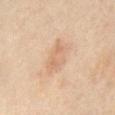notes=total-body-photography surveillance lesion; no biopsy | subject=female, aged 48–52 | image=total-body-photography crop, ~15 mm field of view | anatomic site=the abdomen.